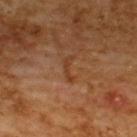<record>
<biopsy_status>not biopsied; imaged during a skin examination</biopsy_status>
<patient>
  <sex>male</sex>
  <age_approx>60</age_approx>
</patient>
<lesion_size>
  <long_diameter_mm_approx>3.0</long_diameter_mm_approx>
</lesion_size>
<image>
  <source>total-body photography crop</source>
  <field_of_view_mm>15</field_of_view_mm>
</image>
<site>back</site>
<lighting>cross-polarized</lighting>
</record>